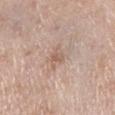Clinical impression:
The lesion was tiled from a total-body skin photograph and was not biopsied.
Acquisition and patient details:
A female patient, aged 68–72. Located on the left lower leg. Cropped from a whole-body photographic skin survey; the tile spans about 15 mm.